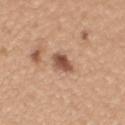follow-up = imaged on a skin check; not biopsied | lighting = white-light | size = ≈3 mm | automated lesion analysis = a footprint of about 4.5 mm²; an automated nevus-likeness rating near 90 out of 100 and a detector confidence of about 100 out of 100 that the crop contains a lesion | patient = female, about 30 years old | location = the left upper arm | image source = ~15 mm crop, total-body skin-cancer survey.